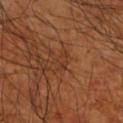This lesion was catalogued during total-body skin photography and was not selected for biopsy.
On the right upper arm.
Imaged with cross-polarized lighting.
A close-up tile cropped from a whole-body skin photograph, about 15 mm across.
Approximately 3 mm at its widest.
The patient is a male aged around 65.